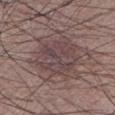Captured during whole-body skin photography for melanoma surveillance; the lesion was not biopsied. The lesion is located on the leg. Longest diameter approximately 6 mm. A male subject, in their mid- to late 70s. A 15 mm crop from a total-body photograph taken for skin-cancer surveillance.